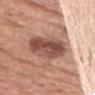| key | value |
|---|---|
| biopsy status | total-body-photography surveillance lesion; no biopsy |
| body site | the head or neck |
| image source | ~15 mm tile from a whole-body skin photo |
| patient | male, aged around 40 |
| image-analysis metrics | a lesion area of about 20 mm² and a shape eccentricity near 0.7; a mean CIELAB color near L≈51 a*≈22 b*≈26 and about 14 CIELAB-L* units darker than the surrounding skin; border irregularity of about 2 on a 0–10 scale and a within-lesion color-variation index near 7/10 |
| lesion size | ~6 mm (longest diameter) |